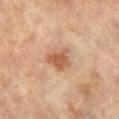follow-up: total-body-photography surveillance lesion; no biopsy
subject: female, roughly 65 years of age
automated metrics: an area of roughly 7 mm² and a shape-asymmetry score of about 0.3 (0 = symmetric); roughly 11 lightness units darker than nearby skin and a normalized border contrast of about 8; a border-irregularity rating of about 3/10 and a peripheral color-asymmetry measure near 0.5
lesion size: about 3.5 mm
anatomic site: the left leg
image source: ~15 mm tile from a whole-body skin photo